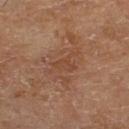| field | value |
|---|---|
| biopsy status | total-body-photography surveillance lesion; no biopsy |
| imaging modality | ~15 mm crop, total-body skin-cancer survey |
| tile lighting | cross-polarized illumination |
| location | the right lower leg |
| patient | male, roughly 65 years of age |